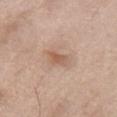imaging modality = ~15 mm tile from a whole-body skin photo; location = the chest; diameter = ≈3 mm; patient = female, aged 73 to 77; tile lighting = white-light.